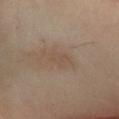Notes:
* notes · catalogued during a skin exam; not biopsied
* body site · the right lower leg
* acquisition · ~15 mm crop, total-body skin-cancer survey
* tile lighting · cross-polarized illumination
* patient · male, aged 53 to 57
* lesion diameter · about 3.5 mm